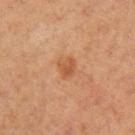biopsy_status: not biopsied; imaged during a skin examination
automated_metrics:
  area_mm2_approx: 4.5
  eccentricity: 0.55
  shape_asymmetry: 0.35
  nevus_likeness_0_100: 25
  lesion_detection_confidence_0_100: 100
image:
  source: total-body photography crop
  field_of_view_mm: 15
site: arm
patient:
  sex: female
  age_approx: 40